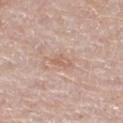Impression: The lesion was photographed on a routine skin check and not biopsied; there is no pathology result. Acquisition and patient details: On the leg. Cropped from a whole-body photographic skin survey; the tile spans about 15 mm. A female subject about 60 years old.